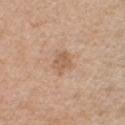Case summary:
• notes: total-body-photography surveillance lesion; no biopsy
• location: the chest
• subject: male, about 55 years old
• diameter: ~3 mm (longest diameter)
• tile lighting: white-light
• image: ~15 mm crop, total-body skin-cancer survey
• automated lesion analysis: a lesion area of about 4.5 mm², an outline eccentricity of about 0.65 (0 = round, 1 = elongated), and a shape-asymmetry score of about 0.25 (0 = symmetric); an average lesion color of about L≈59 a*≈19 b*≈32 (CIELAB); a border-irregularity index near 2.5/10, a within-lesion color-variation index near 2/10, and peripheral color asymmetry of about 0.5; an automated nevus-likeness rating near 10 out of 100 and a detector confidence of about 100 out of 100 that the crop contains a lesion